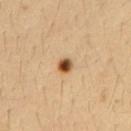biopsy status: imaged on a skin check; not biopsied | subject: female, in their mid- to late 30s | site: the upper back | illumination: cross-polarized | image source: ~15 mm crop, total-body skin-cancer survey.